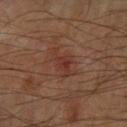Recorded during total-body skin imaging; not selected for excision or biopsy. Longest diameter approximately 4 mm. Located on the right forearm. A region of skin cropped from a whole-body photographic capture, roughly 15 mm wide. The subject is a male aged around 65.